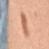Q: Was a biopsy performed?
A: catalogued during a skin exam; not biopsied
Q: How was this image acquired?
A: ~15 mm tile from a whole-body skin photo
Q: Lesion size?
A: ~5.5 mm (longest diameter)
Q: Where on the body is the lesion?
A: the chest
Q: Patient demographics?
A: female, roughly 45 years of age
Q: Illumination type?
A: white-light